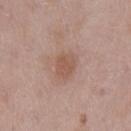Imaged during a routine full-body skin examination; the lesion was not biopsied and no histopathology is available.
A 15 mm close-up tile from a total-body photography series done for melanoma screening.
Measured at roughly 3 mm in maximum diameter.
The tile uses white-light illumination.
From the mid back.
The subject is a male aged 53 to 57.
The total-body-photography lesion software estimated an area of roughly 5.5 mm², a shape eccentricity near 0.65, and two-axis asymmetry of about 0.15. The analysis additionally found a mean CIELAB color near L≈54 a*≈20 b*≈27, a lesion–skin lightness drop of about 8, and a lesion-to-skin contrast of about 6 (normalized; higher = more distinct). The analysis additionally found a classifier nevus-likeness of about 30/100 and a detector confidence of about 100 out of 100 that the crop contains a lesion.